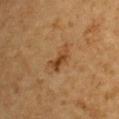  biopsy_status: not biopsied; imaged during a skin examination
  site: upper back
  patient:
    sex: male
    age_approx: 85+
  lesion_size:
    long_diameter_mm_approx: 3.5
  image:
    source: total-body photography crop
    field_of_view_mm: 15
  lighting: cross-polarized